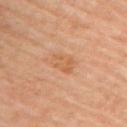Notes:
– workup: no biopsy performed (imaged during a skin exam)
– image source: total-body-photography crop, ~15 mm field of view
– lesion diameter: ~3 mm (longest diameter)
– patient: female, aged 58–62
– illumination: cross-polarized illumination
– site: the back
– TBP lesion metrics: an area of roughly 5 mm², an outline eccentricity of about 0.75 (0 = round, 1 = elongated), and two-axis asymmetry of about 0.25; a lesion color around L≈62 a*≈23 b*≈39 in CIELAB, a lesion–skin lightness drop of about 7, and a normalized border contrast of about 5.5